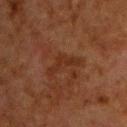{
  "automated_metrics": {
    "area_mm2_approx": 4.5,
    "eccentricity": 0.95,
    "shape_asymmetry": 0.7,
    "vs_skin_contrast_norm": 6.5,
    "border_irregularity_0_10": 8.5,
    "peripheral_color_asymmetry": 0.0,
    "lesion_detection_confidence_0_100": 100
  },
  "patient": {
    "sex": "male",
    "age_approx": 65
  },
  "site": "upper back",
  "lighting": "cross-polarized",
  "image": {
    "source": "total-body photography crop",
    "field_of_view_mm": 15
  },
  "lesion_size": {
    "long_diameter_mm_approx": 4.5
  }
}